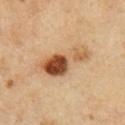Captured during whole-body skin photography for melanoma surveillance; the lesion was not biopsied.
From the right upper arm.
A 15 mm close-up extracted from a 3D total-body photography capture.
Longest diameter approximately 7 mm.
Automated image analysis of the tile measured a border-irregularity index near 4.5/10 and peripheral color asymmetry of about 4.
A male patient aged approximately 55.
Captured under cross-polarized illumination.